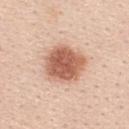The lesion's longest dimension is about 5 mm. A female subject aged around 40. This is a white-light tile. On the upper back. A close-up tile cropped from a whole-body skin photograph, about 15 mm across.